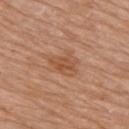Case summary:
* workup · imaged on a skin check; not biopsied
* anatomic site · the right upper arm
* lesion diameter · ~3.5 mm (longest diameter)
* lighting · white-light illumination
* image · 15 mm crop, total-body photography
* patient · female, in their mid-70s